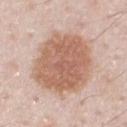No biopsy was performed on this lesion — it was imaged during a full skin examination and was not determined to be concerning. A male subject, aged approximately 60. About 8 mm across. A 15 mm close-up tile from a total-body photography series done for melanoma screening. The tile uses white-light illumination. The total-body-photography lesion software estimated a lesion area of about 42 mm² and two-axis asymmetry of about 0.15. And it measured a color-variation rating of about 4/10 and a peripheral color-asymmetry measure near 1.5. On the chest.